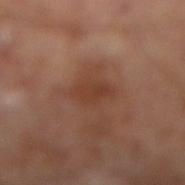• workup · imaged on a skin check; not biopsied
• acquisition · ~15 mm crop, total-body skin-cancer survey
• location · the right lower leg
• size · ~3.5 mm (longest diameter)
• image-analysis metrics · an area of roughly 6.5 mm² and an eccentricity of roughly 0.65; a classifier nevus-likeness of about 10/100 and a detector confidence of about 100 out of 100 that the crop contains a lesion